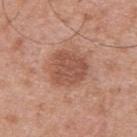notes: catalogued during a skin exam; not biopsied | subject: male, roughly 30 years of age | anatomic site: the upper back | image: ~15 mm crop, total-body skin-cancer survey | lighting: white-light | diameter: ~4.5 mm (longest diameter).